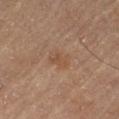This is a cross-polarized tile. About 3 mm across. A lesion tile, about 15 mm wide, cut from a 3D total-body photograph. The lesion is located on the leg. A male subject aged approximately 70.